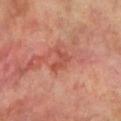Assessment: Captured during whole-body skin photography for melanoma surveillance; the lesion was not biopsied. Image and clinical context: Cropped from a whole-body photographic skin survey; the tile spans about 15 mm. Longest diameter approximately 2.5 mm. A male subject, aged approximately 70. The lesion is on the left lower leg.